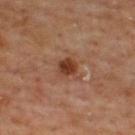Part of a total-body skin-imaging series; this lesion was reviewed on a skin check and was not flagged for biopsy.
Automated image analysis of the tile measured about 11 CIELAB-L* units darker than the surrounding skin. It also reported border irregularity of about 1.5 on a 0–10 scale, a color-variation rating of about 3/10, and peripheral color asymmetry of about 1.
A 15 mm crop from a total-body photograph taken for skin-cancer surveillance.
A male patient about 65 years old.
On the upper back.